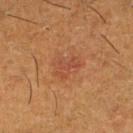Context: A 15 mm crop from a total-body photograph taken for skin-cancer surveillance. The lesion-visualizer software estimated a footprint of about 7.5 mm², an eccentricity of roughly 0.65, and a symmetry-axis asymmetry near 0.35. The analysis additionally found an average lesion color of about L≈46 a*≈25 b*≈33 (CIELAB) and a normalized border contrast of about 5. The software also gave a detector confidence of about 100 out of 100 that the crop contains a lesion. A male subject about 60 years old. The lesion is located on the right lower leg. This is a cross-polarized tile.